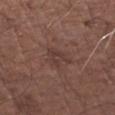Q: Is there a histopathology result?
A: catalogued during a skin exam; not biopsied
Q: Where on the body is the lesion?
A: the left forearm
Q: Illumination type?
A: white-light
Q: Who is the patient?
A: male, roughly 75 years of age
Q: What kind of image is this?
A: ~15 mm tile from a whole-body skin photo
Q: What is the lesion's diameter?
A: about 3.5 mm